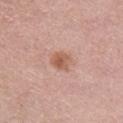* follow-up — total-body-photography surveillance lesion; no biopsy
* subject — female, roughly 50 years of age
* anatomic site — the front of the torso
* diameter — ≈2.5 mm
* image source — total-body-photography crop, ~15 mm field of view